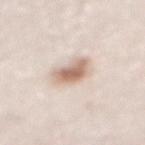No biopsy was performed on this lesion — it was imaged during a full skin examination and was not determined to be concerning.
The total-body-photography lesion software estimated an area of roughly 8.5 mm², a shape eccentricity near 0.55, and a symmetry-axis asymmetry near 0.25. The software also gave an average lesion color of about L≈68 a*≈16 b*≈27 (CIELAB), about 14 CIELAB-L* units darker than the surrounding skin, and a lesion-to-skin contrast of about 8.5 (normalized; higher = more distinct). And it measured a border-irregularity index near 2.5/10, a within-lesion color-variation index near 5.5/10, and a peripheral color-asymmetry measure near 1.5. The analysis additionally found an automated nevus-likeness rating near 100 out of 100.
Cropped from a total-body skin-imaging series; the visible field is about 15 mm.
A male patient, about 80 years old.
The lesion is on the front of the torso.
Imaged with white-light lighting.